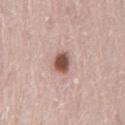Imaged during a routine full-body skin examination; the lesion was not biopsied and no histopathology is available. Measured at roughly 3 mm in maximum diameter. A 15 mm crop from a total-body photograph taken for skin-cancer surveillance. From the lower back. Automated image analysis of the tile measured an eccentricity of roughly 0.65 and a symmetry-axis asymmetry near 0.2. The analysis additionally found an average lesion color of about L≈52 a*≈21 b*≈25 (CIELAB) and about 18 CIELAB-L* units darker than the surrounding skin. And it measured a classifier nevus-likeness of about 95/100. Captured under white-light illumination. A male patient, aged around 75.